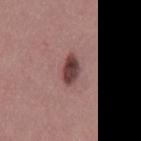This lesion was catalogued during total-body skin photography and was not selected for biopsy. A male patient in their 40s. The recorded lesion diameter is about 3.5 mm. This is a white-light tile. Automated image analysis of the tile measured an outline eccentricity of about 0.75 (0 = round, 1 = elongated). The software also gave a mean CIELAB color near L≈41 a*≈21 b*≈19, roughly 15 lightness units darker than nearby skin, and a normalized border contrast of about 11.5. And it measured a classifier nevus-likeness of about 95/100 and a detector confidence of about 100 out of 100 that the crop contains a lesion. Cropped from a whole-body photographic skin survey; the tile spans about 15 mm. On the mid back.